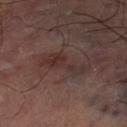{"site": "left thigh", "automated_metrics": {"eccentricity": 0.95, "nevus_likeness_0_100": 0, "lesion_detection_confidence_0_100": 90}, "lesion_size": {"long_diameter_mm_approx": 5.5}, "lighting": "cross-polarized", "image": {"source": "total-body photography crop", "field_of_view_mm": 15}}